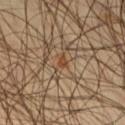{"biopsy_status": "not biopsied; imaged during a skin examination", "lighting": "cross-polarized", "automated_metrics": {"cielab_L": 43, "cielab_a": 19, "cielab_b": 33, "vs_skin_darker_L": 7.0, "vs_skin_contrast_norm": 7.5, "border_irregularity_0_10": 3.0, "color_variation_0_10": 0.0, "peripheral_color_asymmetry": 0.0, "nevus_likeness_0_100": 5, "lesion_detection_confidence_0_100": 90}, "image": {"source": "total-body photography crop", "field_of_view_mm": 15}, "lesion_size": {"long_diameter_mm_approx": 1.5}, "site": "right thigh", "patient": {"sex": "male", "age_approx": 45}}